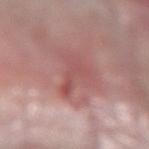No biopsy was performed on this lesion — it was imaged during a full skin examination and was not determined to be concerning. A roughly 15 mm field-of-view crop from a total-body skin photograph. The patient is a male about 75 years old. This is a white-light tile. An algorithmic analysis of the crop reported a shape eccentricity near 0.95 and two-axis asymmetry of about 0.6. Longest diameter approximately 5 mm. On the left forearm.